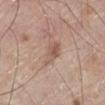Q: Was this lesion biopsied?
A: no biopsy performed (imaged during a skin exam)
Q: What is the imaging modality?
A: ~15 mm crop, total-body skin-cancer survey
Q: How was the tile lit?
A: white-light illumination
Q: Lesion location?
A: the right leg
Q: What is the lesion's diameter?
A: ~3 mm (longest diameter)
Q: What are the patient's age and sex?
A: male, aged approximately 80